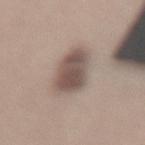<case>
<diagnosis>
  <histopathology>dysplastic (Clark) nevus</histopathology>
  <malignancy>benign</malignancy>
  <taxonomic_path>Benign; Benign melanocytic proliferations; Nevus; Nevus, Atypical, Dysplastic, or Clark</taxonomic_path>
</diagnosis>
</case>